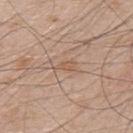biopsy_status: not biopsied; imaged during a skin examination
image:
  source: total-body photography crop
  field_of_view_mm: 15
lighting: white-light
lesion_size:
  long_diameter_mm_approx: 2.5
patient:
  sex: male
  age_approx: 50
site: back